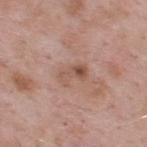This lesion was catalogued during total-body skin photography and was not selected for biopsy.
A lesion tile, about 15 mm wide, cut from a 3D total-body photograph.
Captured under white-light illumination.
The subject is a male in their mid- to late 50s.
An algorithmic analysis of the crop reported a normalized lesion–skin contrast near 6. The analysis additionally found border irregularity of about 4 on a 0–10 scale and radial color variation of about 4.
Located on the upper back.
The lesion's longest dimension is about 3 mm.